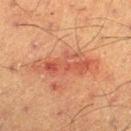{"biopsy_status": "not biopsied; imaged during a skin examination", "image": {"source": "total-body photography crop", "field_of_view_mm": 15}, "site": "right lower leg", "lighting": "cross-polarized", "lesion_size": {"long_diameter_mm_approx": 7.5}, "patient": {"sex": "male", "age_approx": 60}}